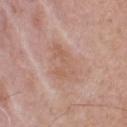{"biopsy_status": "not biopsied; imaged during a skin examination", "image": {"source": "total-body photography crop", "field_of_view_mm": 15}, "site": "upper back", "patient": {"sex": "male", "age_approx": 55}, "lesion_size": {"long_diameter_mm_approx": 5.0}, "lighting": "white-light"}